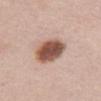Recorded during total-body skin imaging; not selected for excision or biopsy. A female subject, aged approximately 50. On the abdomen. A close-up tile cropped from a whole-body skin photograph, about 15 mm across.